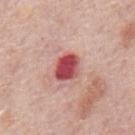The lesion was tiled from a total-body skin photograph and was not biopsied. Located on the mid back. About 3.5 mm across. This image is a 15 mm lesion crop taken from a total-body photograph. The total-body-photography lesion software estimated a classifier nevus-likeness of about 0/100 and a detector confidence of about 100 out of 100 that the crop contains a lesion. A male subject aged around 75. This is a white-light tile.